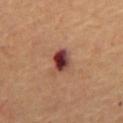* workup — no biopsy performed (imaged during a skin exam)
* site — the chest
* size — about 3.5 mm
* image source — ~15 mm tile from a whole-body skin photo
* subject — male, about 65 years old
* illumination — cross-polarized illumination